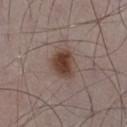A 15 mm close-up extracted from a 3D total-body photography capture.
The recorded lesion diameter is about 4 mm.
A male subject in their 50s.
Imaged with white-light lighting.
The lesion is located on the right thigh.
An algorithmic analysis of the crop reported an outline eccentricity of about 0.65 (0 = round, 1 = elongated). The software also gave a lesion color around L≈41 a*≈17 b*≈23 in CIELAB, roughly 12 lightness units darker than nearby skin, and a normalized lesion–skin contrast near 10.5.